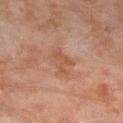biopsy status: imaged on a skin check; not biopsied
image source: total-body-photography crop, ~15 mm field of view
site: the right lower leg
subject: male, aged around 60
lighting: cross-polarized illumination
image-analysis metrics: a mean CIELAB color near L≈50 a*≈22 b*≈30 and a normalized lesion–skin contrast near 5.5; a classifier nevus-likeness of about 0/100 and a detector confidence of about 100 out of 100 that the crop contains a lesion
size: ~3.5 mm (longest diameter)